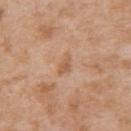Clinical impression: Recorded during total-body skin imaging; not selected for excision or biopsy. Background: A female patient aged approximately 40. A region of skin cropped from a whole-body photographic capture, roughly 15 mm wide. The lesion is on the upper back.